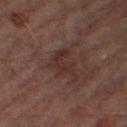Part of a total-body skin-imaging series; this lesion was reviewed on a skin check and was not flagged for biopsy. The subject is a male in their mid- to late 60s. This is a cross-polarized tile. The total-body-photography lesion software estimated a lesion area of about 6.5 mm² and a shape-asymmetry score of about 0.45 (0 = symmetric). It also reported an average lesion color of about L≈28 a*≈18 b*≈20 (CIELAB), a lesion–skin lightness drop of about 6, and a normalized lesion–skin contrast near 6.5. The analysis additionally found border irregularity of about 7 on a 0–10 scale, internal color variation of about 2 on a 0–10 scale, and a peripheral color-asymmetry measure near 1. The software also gave a nevus-likeness score of about 0/100 and lesion-presence confidence of about 100/100. A lesion tile, about 15 mm wide, cut from a 3D total-body photograph. Approximately 4 mm at its widest. On the right thigh.